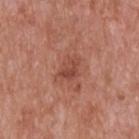  biopsy_status: not biopsied; imaged during a skin examination
  patient:
    sex: male
    age_approx: 60
  lesion_size:
    long_diameter_mm_approx: 3.0
  image:
    source: total-body photography crop
    field_of_view_mm: 15
  lighting: white-light
  site: upper back